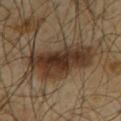Impression: No biopsy was performed on this lesion — it was imaged during a full skin examination and was not determined to be concerning. Background: Approximately 7.5 mm at its widest. A male patient roughly 65 years of age. Automated image analysis of the tile measured a border-irregularity index near 4.5/10 and a within-lesion color-variation index near 5/10. From the upper back. The tile uses cross-polarized illumination. A region of skin cropped from a whole-body photographic capture, roughly 15 mm wide.